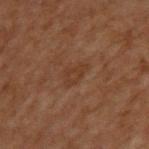biopsy_status: not biopsied; imaged during a skin examination
site: upper back
patient:
  sex: male
  age_approx: 70
image:
  source: total-body photography crop
  field_of_view_mm: 15
lighting: cross-polarized
automated_metrics:
  color_variation_0_10: 1.5
  peripheral_color_asymmetry: 0.5